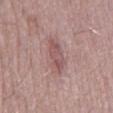Imaged during a routine full-body skin examination; the lesion was not biopsied and no histopathology is available.
A 15 mm close-up extracted from a 3D total-body photography capture.
From the back.
Captured under white-light illumination.
A male patient in their mid- to late 60s.